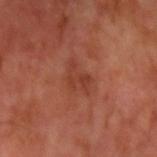workup: no biopsy performed (imaged during a skin exam); size: ~3.5 mm (longest diameter); anatomic site: the left upper arm; acquisition: 15 mm crop, total-body photography; patient: male, aged around 70; automated lesion analysis: a classifier nevus-likeness of about 0/100 and lesion-presence confidence of about 100/100.